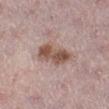biopsy status=catalogued during a skin exam; not biopsied
size=about 5 mm
imaging modality=~15 mm tile from a whole-body skin photo
patient=female, in their mid-40s
site=the left lower leg